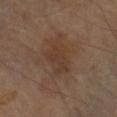Findings:
• follow-up — total-body-photography surveillance lesion; no biopsy
• tile lighting — cross-polarized
• patient — male, aged 63–67
• lesion diameter — about 5 mm
• acquisition — 15 mm crop, total-body photography
• automated lesion analysis — an area of roughly 10 mm², an outline eccentricity of about 0.8 (0 = round, 1 = elongated), and two-axis asymmetry of about 0.35
• site — the arm